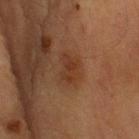Part of a total-body skin-imaging series; this lesion was reviewed on a skin check and was not flagged for biopsy.
A 15 mm close-up extracted from a 3D total-body photography capture.
On the arm.
This is a cross-polarized tile.
Approximately 3.5 mm at its widest.
The subject is a male approximately 85 years of age or older.